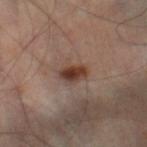biopsy_status: not biopsied; imaged during a skin examination
lighting: cross-polarized
site: left leg
image:
  source: total-body photography crop
  field_of_view_mm: 15
patient:
  sex: male
  age_approx: 50
lesion_size:
  long_diameter_mm_approx: 3.5